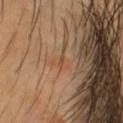Notes:
- biopsy status: total-body-photography surveillance lesion; no biopsy
- image: ~15 mm crop, total-body skin-cancer survey
- lesion size: ≈1.5 mm
- subject: male, about 45 years old
- site: the head or neck